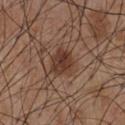Recorded during total-body skin imaging; not selected for excision or biopsy. Cropped from a whole-body photographic skin survey; the tile spans about 15 mm. A male subject about 55 years old. Captured under white-light illumination. An algorithmic analysis of the crop reported an area of roughly 7 mm² and a shape-asymmetry score of about 0.2 (0 = symmetric). It also reported a border-irregularity rating of about 2/10 and radial color variation of about 1.5. About 3.5 mm across. On the chest.